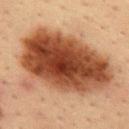Captured during whole-body skin photography for melanoma surveillance; the lesion was not biopsied. Longest diameter approximately 11.5 mm. The lesion is on the back. A 15 mm crop from a total-body photograph taken for skin-cancer surveillance. A male subject, aged 33–37.